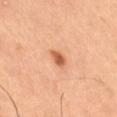Q: What kind of image is this?
A: total-body-photography crop, ~15 mm field of view
Q: What is the anatomic site?
A: the right thigh
Q: What lighting was used for the tile?
A: cross-polarized illumination
Q: Patient demographics?
A: male, about 65 years old
Q: What did automated image analysis measure?
A: a mean CIELAB color near L≈60 a*≈29 b*≈38 and a lesion-to-skin contrast of about 9 (normalized; higher = more distinct); a classifier nevus-likeness of about 100/100 and a detector confidence of about 100 out of 100 that the crop contains a lesion